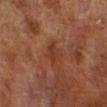Clinical impression:
No biopsy was performed on this lesion — it was imaged during a full skin examination and was not determined to be concerning.
Image and clinical context:
The lesion is on the right lower leg. Cropped from a total-body skin-imaging series; the visible field is about 15 mm. A male patient, about 70 years old.